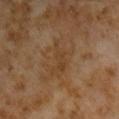No biopsy was performed on this lesion — it was imaged during a full skin examination and was not determined to be concerning. Captured under cross-polarized illumination. The lesion is located on the right upper arm. Cropped from a whole-body photographic skin survey; the tile spans about 15 mm. Automated tile analysis of the lesion measured a footprint of about 5 mm² and two-axis asymmetry of about 0.55. It also reported a lesion color around L≈37 a*≈16 b*≈31 in CIELAB and a lesion–skin lightness drop of about 5. It also reported a nevus-likeness score of about 0/100. A male subject roughly 60 years of age.